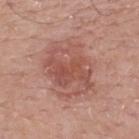Cropped from a whole-body photographic skin survey; the tile spans about 15 mm.
The tile uses white-light illumination.
From the mid back.
About 6 mm across.
A male patient, roughly 75 years of age.